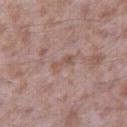Captured during whole-body skin photography for melanoma surveillance; the lesion was not biopsied. Captured under white-light illumination. A lesion tile, about 15 mm wide, cut from a 3D total-body photograph. On the right thigh. The subject is a male approximately 45 years of age. The recorded lesion diameter is about 3.5 mm.